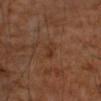anatomic site: the right upper arm
patient: male, aged 58–62
size: ≈2.5 mm
imaging modality: total-body-photography crop, ~15 mm field of view
automated lesion analysis: a footprint of about 3 mm², a shape eccentricity near 0.75, and a shape-asymmetry score of about 0.45 (0 = symmetric); a mean CIELAB color near L≈31 a*≈18 b*≈27, roughly 5 lightness units darker than nearby skin, and a lesion-to-skin contrast of about 5.5 (normalized; higher = more distinct); a nevus-likeness score of about 0/100 and a lesion-detection confidence of about 100/100